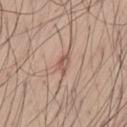<case>
  <biopsy_status>not biopsied; imaged during a skin examination</biopsy_status>
  <patient>
    <sex>male</sex>
    <age_approx>55</age_approx>
  </patient>
  <image>
    <source>total-body photography crop</source>
    <field_of_view_mm>15</field_of_view_mm>
  </image>
  <lighting>white-light</lighting>
  <site>lower back</site>
  <lesion_size>
    <long_diameter_mm_approx>2.5</long_diameter_mm_approx>
  </lesion_size>
</case>